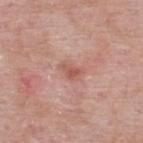  biopsy_status: not biopsied; imaged during a skin examination
  site: upper back
  image:
    source: total-body photography crop
    field_of_view_mm: 15
  lesion_size:
    long_diameter_mm_approx: 2.5
  patient:
    sex: male
    age_approx: 55
  lighting: white-light